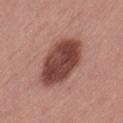Imaged during a routine full-body skin examination; the lesion was not biopsied and no histopathology is available.
A female subject, aged 53 to 57.
Captured under white-light illumination.
A 15 mm crop from a total-body photograph taken for skin-cancer surveillance.
About 6.5 mm across.
The lesion is on the left thigh.
The lesion-visualizer software estimated a lesion area of about 23 mm² and an outline eccentricity of about 0.75 (0 = round, 1 = elongated). The software also gave about 16 CIELAB-L* units darker than the surrounding skin and a lesion-to-skin contrast of about 11.5 (normalized; higher = more distinct).